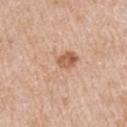biopsy status: no biopsy performed (imaged during a skin exam); anatomic site: the arm; patient: male, aged approximately 65; illumination: white-light illumination; image: total-body-photography crop, ~15 mm field of view.